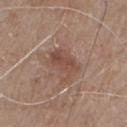{
  "biopsy_status": "not biopsied; imaged during a skin examination"
}